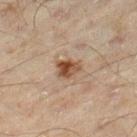Imaged during a routine full-body skin examination; the lesion was not biopsied and no histopathology is available. A male subject, aged 43–47. Located on the leg. This image is a 15 mm lesion crop taken from a total-body photograph.